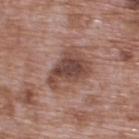Captured during whole-body skin photography for melanoma surveillance; the lesion was not biopsied.
The lesion is on the upper back.
A roughly 15 mm field-of-view crop from a total-body skin photograph.
Longest diameter approximately 5.5 mm.
Automated tile analysis of the lesion measured an area of roughly 16 mm² and two-axis asymmetry of about 0.35. It also reported a border-irregularity index near 4.5/10 and internal color variation of about 5 on a 0–10 scale.
A male patient, aged 68–72.